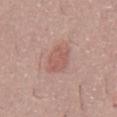Background: The lesion's longest dimension is about 3.5 mm. From the front of the torso. A male patient, aged approximately 45. A 15 mm close-up extracted from a 3D total-body photography capture. Captured under white-light illumination.